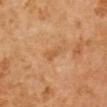  biopsy_status: not biopsied; imaged during a skin examination
  patient:
    sex: male
    age_approx: 60
  lesion_size:
    long_diameter_mm_approx: 3.0
  site: right arm
  image:
    source: total-body photography crop
    field_of_view_mm: 15